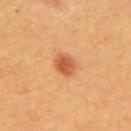The lesion was tiled from a total-body skin photograph and was not biopsied. Longest diameter approximately 3 mm. This is a cross-polarized tile. A female patient aged 58–62. On the upper back. Cropped from a whole-body photographic skin survey; the tile spans about 15 mm. The lesion-visualizer software estimated a lesion–skin lightness drop of about 13 and a normalized lesion–skin contrast near 8. The software also gave a nevus-likeness score of about 95/100.